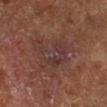Recorded during total-body skin imaging; not selected for excision or biopsy.
Imaged with cross-polarized lighting.
The lesion is located on the right lower leg.
A roughly 15 mm field-of-view crop from a total-body skin photograph.
A patient about 65 years old.
Measured at roughly 6 mm in maximum diameter.